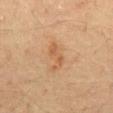biopsy_status: not biopsied; imaged during a skin examination
lighting: cross-polarized
patient:
  sex: male
  age_approx: 60
image:
  source: total-body photography crop
  field_of_view_mm: 15
site: abdomen